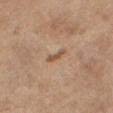This lesion was catalogued during total-body skin photography and was not selected for biopsy. A lesion tile, about 15 mm wide, cut from a 3D total-body photograph. The patient is a female roughly 60 years of age. The lesion is located on the mid back. The lesion-visualizer software estimated roughly 10 lightness units darker than nearby skin and a lesion-to-skin contrast of about 7 (normalized; higher = more distinct). The tile uses white-light illumination. Longest diameter approximately 3 mm.